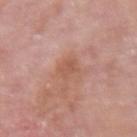Recorded during total-body skin imaging; not selected for excision or biopsy.
A female subject, aged 63 to 67.
This image is a 15 mm lesion crop taken from a total-body photograph.
The lesion is on the right upper arm.